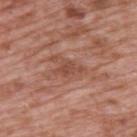This lesion was catalogued during total-body skin photography and was not selected for biopsy. The subject is a male aged around 70. Imaged with white-light lighting. Longest diameter approximately 3.5 mm. A lesion tile, about 15 mm wide, cut from a 3D total-body photograph. Automated image analysis of the tile measured a footprint of about 4.5 mm² and a symmetry-axis asymmetry near 0.35. The analysis additionally found about 8 CIELAB-L* units darker than the surrounding skin and a lesion-to-skin contrast of about 6 (normalized; higher = more distinct). The software also gave a nevus-likeness score of about 0/100 and a lesion-detection confidence of about 70/100. From the back.